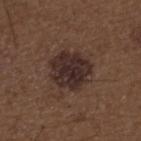diameter = ~5 mm (longest diameter); site = the back; patient = male, aged 48 to 52; image source = total-body-photography crop, ~15 mm field of view.